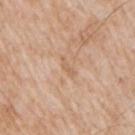  biopsy_status: not biopsied; imaged during a skin examination
  image:
    source: total-body photography crop
    field_of_view_mm: 15
  site: right upper arm
  lesion_size:
    long_diameter_mm_approx: 2.5
  lighting: white-light
  patient:
    sex: male
    age_approx: 65